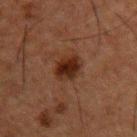biopsy status — total-body-photography surveillance lesion; no biopsy | subject — male, approximately 50 years of age | anatomic site — the back | image — ~15 mm crop, total-body skin-cancer survey.